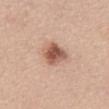Assessment:
No biopsy was performed on this lesion — it was imaged during a full skin examination and was not determined to be concerning.
Clinical summary:
The tile uses white-light illumination. The subject is a female about 45 years old. Approximately 3.5 mm at its widest. A 15 mm crop from a total-body photograph taken for skin-cancer surveillance. From the abdomen. An algorithmic analysis of the crop reported an eccentricity of roughly 0.5. The software also gave a border-irregularity rating of about 2/10 and radial color variation of about 1.5. And it measured an automated nevus-likeness rating near 95 out of 100.